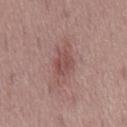The lesion was tiled from a total-body skin photograph and was not biopsied.